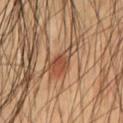biopsy_status: not biopsied; imaged during a skin examination
automated_metrics:
  area_mm2_approx: 4.0
  eccentricity: 0.85
  shape_asymmetry: 0.25
  cielab_L: 45
  cielab_a: 23
  cielab_b: 31
  vs_skin_darker_L: 9.0
  nevus_likeness_0_100: 100
  lesion_detection_confidence_0_100: 95
site: chest
lighting: cross-polarized
patient:
  sex: male
  age_approx: 55
image:
  source: total-body photography crop
  field_of_view_mm: 15